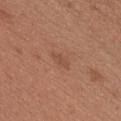Case summary:
• follow-up: no biopsy performed (imaged during a skin exam)
• tile lighting: white-light illumination
• image: total-body-photography crop, ~15 mm field of view
• lesion size: about 2.5 mm
• subject: male, aged 33–37
• anatomic site: the chest